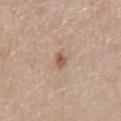Q: Is there a histopathology result?
A: no biopsy performed (imaged during a skin exam)
Q: What are the patient's age and sex?
A: male, aged 58 to 62
Q: What kind of image is this?
A: 15 mm crop, total-body photography
Q: Lesion location?
A: the mid back
Q: Illumination type?
A: white-light illumination